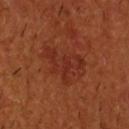workup=catalogued during a skin exam; not biopsied | illumination=cross-polarized | image=~15 mm tile from a whole-body skin photo | patient=male, aged 53 to 57 | location=the head or neck | automated lesion analysis=a footprint of about 12 mm² and an eccentricity of roughly 0.8; a mean CIELAB color near L≈28 a*≈27 b*≈29 and a lesion–skin lightness drop of about 5.